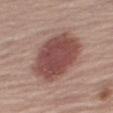Case summary:
* workup — imaged on a skin check; not biopsied
* image — ~15 mm crop, total-body skin-cancer survey
* site — the right thigh
* patient — female, aged 63–67
* TBP lesion metrics — a footprint of about 30 mm², an eccentricity of roughly 0.8, and a shape-asymmetry score of about 0.1 (0 = symmetric); a lesion color around L≈47 a*≈22 b*≈23 in CIELAB, a lesion–skin lightness drop of about 14, and a normalized border contrast of about 10
* lesion diameter — ≈7.5 mm